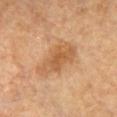  biopsy_status: not biopsied; imaged during a skin examination
  image:
    source: total-body photography crop
    field_of_view_mm: 15
  site: chest
  patient:
    sex: female
    age_approx: 60
  lighting: cross-polarized